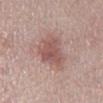Clinical impression: Captured during whole-body skin photography for melanoma surveillance; the lesion was not biopsied. Context: A 15 mm close-up tile from a total-body photography series done for melanoma screening. The subject is a male aged approximately 70. The lesion is located on the mid back.